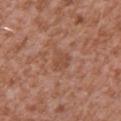notes: no biopsy performed (imaged during a skin exam) | size: ≈3 mm | image: ~15 mm tile from a whole-body skin photo | location: the chest | tile lighting: white-light illumination | patient: male, in their mid-40s | image-analysis metrics: a lesion area of about 5 mm² and an eccentricity of roughly 0.45; an average lesion color of about L≈49 a*≈22 b*≈30 (CIELAB), roughly 6 lightness units darker than nearby skin, and a normalized border contrast of about 5; a classifier nevus-likeness of about 0/100.